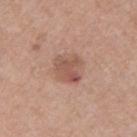notes: no biopsy performed (imaged during a skin exam) | subject: male, about 55 years old | image source: ~15 mm crop, total-body skin-cancer survey | location: the left upper arm.